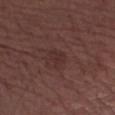Findings:
– workup — imaged on a skin check; not biopsied
– subject — male, in their mid-70s
– lesion diameter — ~2.5 mm (longest diameter)
– anatomic site — the right forearm
– tile lighting — white-light illumination
– automated metrics — a lesion area of about 4 mm², an outline eccentricity of about 0.7 (0 = round, 1 = elongated), and a shape-asymmetry score of about 0.3 (0 = symmetric); a lesion–skin lightness drop of about 5 and a lesion-to-skin contrast of about 5 (normalized; higher = more distinct); a border-irregularity rating of about 3/10, internal color variation of about 1.5 on a 0–10 scale, and a peripheral color-asymmetry measure near 0.5; a classifier nevus-likeness of about 0/100 and a lesion-detection confidence of about 100/100
– imaging modality — ~15 mm tile from a whole-body skin photo